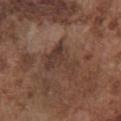follow-up: catalogued during a skin exam; not biopsied
subject: male, approximately 75 years of age
imaging modality: 15 mm crop, total-body photography
illumination: white-light illumination
size: ~4.5 mm (longest diameter)
location: the chest